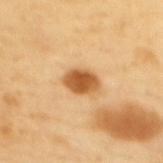  biopsy_status: not biopsied; imaged during a skin examination
  image:
    source: total-body photography crop
    field_of_view_mm: 15
  site: mid back
  lesion_size:
    long_diameter_mm_approx: 3.5
  patient:
    sex: female
    age_approx: 55